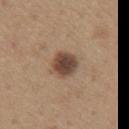workup: total-body-photography surveillance lesion; no biopsy | body site: the front of the torso | tile lighting: white-light | patient: male, about 65 years old | imaging modality: total-body-photography crop, ~15 mm field of view.